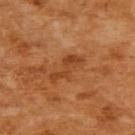Q: Lesion size?
A: ~4.5 mm (longest diameter)
Q: Who is the patient?
A: female, aged 53–57
Q: What kind of image is this?
A: ~15 mm tile from a whole-body skin photo
Q: Lesion location?
A: the upper back
Q: Automated lesion metrics?
A: an area of roughly 6 mm² and a shape-asymmetry score of about 0.6 (0 = symmetric)